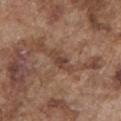Recorded during total-body skin imaging; not selected for excision or biopsy.
Cropped from a whole-body photographic skin survey; the tile spans about 15 mm.
A male patient, in their mid-70s.
From the chest.